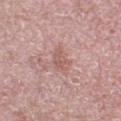workup=catalogued during a skin exam; not biopsied | automated lesion analysis=a within-lesion color-variation index near 1.5/10 | subject=female, in their 40s | size=about 3 mm | body site=the right thigh | image=total-body-photography crop, ~15 mm field of view.